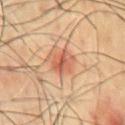Assessment:
This lesion was catalogued during total-body skin photography and was not selected for biopsy.